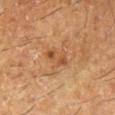{
  "biopsy_status": "not biopsied; imaged during a skin examination",
  "patient": {
    "sex": "male",
    "age_approx": 60
  },
  "site": "left lower leg",
  "automated_metrics": {
    "cielab_L": 49,
    "cielab_a": 22,
    "cielab_b": 36,
    "vs_skin_darker_L": 8.0,
    "vs_skin_contrast_norm": 6.0,
    "border_irregularity_0_10": 3.0
  },
  "lesion_size": {
    "long_diameter_mm_approx": 3.5
  },
  "image": {
    "source": "total-body photography crop",
    "field_of_view_mm": 15
  }
}